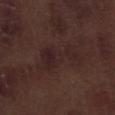<case>
<biopsy_status>not biopsied; imaged during a skin examination</biopsy_status>
<site>right lower leg</site>
<image>
  <source>total-body photography crop</source>
  <field_of_view_mm>15</field_of_view_mm>
</image>
<lesion_size>
  <long_diameter_mm_approx>6.5</long_diameter_mm_approx>
</lesion_size>
<automated_metrics>
  <shape_asymmetry>0.5</shape_asymmetry>
  <vs_skin_darker_L>4.0</vs_skin_darker_L>
</automated_metrics>
<lighting>white-light</lighting>
<patient>
  <sex>male</sex>
  <age_approx>70</age_approx>
</patient>
</case>